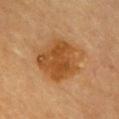Part of a total-body skin-imaging series; this lesion was reviewed on a skin check and was not flagged for biopsy. The total-body-photography lesion software estimated an average lesion color of about L≈44 a*≈21 b*≈37 (CIELAB) and about 9 CIELAB-L* units darker than the surrounding skin. The software also gave a border-irregularity index near 2.5/10, internal color variation of about 4.5 on a 0–10 scale, and a peripheral color-asymmetry measure near 1.5. And it measured a classifier nevus-likeness of about 75/100 and lesion-presence confidence of about 100/100. Imaged with cross-polarized lighting. Approximately 6 mm at its widest. Cropped from a whole-body photographic skin survey; the tile spans about 15 mm. Located on the chest. The patient is a female aged around 60.